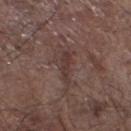– notes — catalogued during a skin exam; not biopsied
– tile lighting — white-light illumination
– image-analysis metrics — a lesion area of about 5.5 mm², an outline eccentricity of about 0.9 (0 = round, 1 = elongated), and a shape-asymmetry score of about 0.45 (0 = symmetric); a mean CIELAB color near L≈36 a*≈17 b*≈19 and a lesion–skin lightness drop of about 7; a border-irregularity rating of about 5/10, internal color variation of about 1.5 on a 0–10 scale, and peripheral color asymmetry of about 0.5; a nevus-likeness score of about 0/100 and a detector confidence of about 70 out of 100 that the crop contains a lesion
– lesion size — ≈4 mm
– anatomic site — the left lower leg
– patient — male, about 80 years old
– imaging modality — ~15 mm tile from a whole-body skin photo